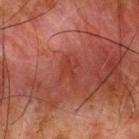Assessment:
This lesion was catalogued during total-body skin photography and was not selected for biopsy.
Context:
A male subject, approximately 65 years of age. The lesion is on the upper back. A roughly 15 mm field-of-view crop from a total-body skin photograph.